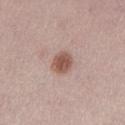workup=catalogued during a skin exam; not biopsied